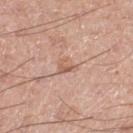The lesion was photographed on a routine skin check and not biopsied; there is no pathology result. A male patient approximately 55 years of age. Cropped from a total-body skin-imaging series; the visible field is about 15 mm. The total-body-photography lesion software estimated a footprint of about 3.5 mm², an outline eccentricity of about 0.65 (0 = round, 1 = elongated), and a shape-asymmetry score of about 0.4 (0 = symmetric). It also reported an average lesion color of about L≈60 a*≈20 b*≈29 (CIELAB), about 9 CIELAB-L* units darker than the surrounding skin, and a normalized border contrast of about 6. It also reported border irregularity of about 3.5 on a 0–10 scale and a color-variation rating of about 2.5/10. The analysis additionally found a classifier nevus-likeness of about 0/100. The lesion is on the left thigh. Captured under white-light illumination.